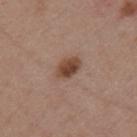Q: Is there a histopathology result?
A: imaged on a skin check; not biopsied
Q: What lighting was used for the tile?
A: white-light illumination
Q: Lesion location?
A: the mid back
Q: How large is the lesion?
A: about 3 mm
Q: What is the imaging modality?
A: ~15 mm tile from a whole-body skin photo
Q: Who is the patient?
A: female, aged approximately 45